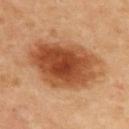Imaged during a routine full-body skin examination; the lesion was not biopsied and no histopathology is available. A 15 mm close-up tile from a total-body photography series done for melanoma screening. The recorded lesion diameter is about 9.5 mm. The total-body-photography lesion software estimated a border-irregularity index near 2.5/10, internal color variation of about 7.5 on a 0–10 scale, and a peripheral color-asymmetry measure near 2. The lesion is located on the upper back. A male subject aged 48 to 52.